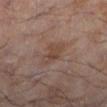Captured during whole-body skin photography for melanoma surveillance; the lesion was not biopsied.
Measured at roughly 3 mm in maximum diameter.
From the left lower leg.
A male patient aged 53 to 57.
This image is a 15 mm lesion crop taken from a total-body photograph.